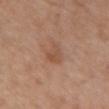  automated_metrics:
    cielab_L: 51
    cielab_a: 20
    cielab_b: 30
    vs_skin_contrast_norm: 5.0
    border_irregularity_0_10: 3.0
    color_variation_0_10: 2.5
    peripheral_color_asymmetry: 0.5
    nevus_likeness_0_100: 30
    lesion_detection_confidence_0_100: 100
  site: chest
  lesion_size:
    long_diameter_mm_approx: 3.0
  image:
    source: total-body photography crop
    field_of_view_mm: 15
  patient:
    sex: female
    age_approx: 65